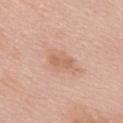{
  "lesion_size": {
    "long_diameter_mm_approx": 3.0
  },
  "site": "mid back",
  "patient": {
    "sex": "female",
    "age_approx": 60
  },
  "lighting": "white-light",
  "automated_metrics": {
    "border_irregularity_0_10": 2.5,
    "color_variation_0_10": 1.5,
    "peripheral_color_asymmetry": 0.5,
    "nevus_likeness_0_100": 5
  },
  "image": {
    "source": "total-body photography crop",
    "field_of_view_mm": 15
  }
}